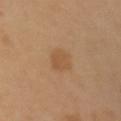{
  "site": "left upper arm",
  "patient": {
    "sex": "female",
    "age_approx": 55
  },
  "lighting": "cross-polarized",
  "lesion_size": {
    "long_diameter_mm_approx": 3.5
  },
  "image": {
    "source": "total-body photography crop",
    "field_of_view_mm": 15
  }
}